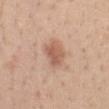Q: Was this lesion biopsied?
A: total-body-photography surveillance lesion; no biopsy
Q: How was this image acquired?
A: ~15 mm tile from a whole-body skin photo
Q: Who is the patient?
A: female, about 30 years old
Q: What did automated image analysis measure?
A: a nevus-likeness score of about 80/100
Q: What is the anatomic site?
A: the chest